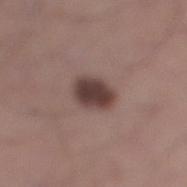Clinical impression: This lesion was catalogued during total-body skin photography and was not selected for biopsy. Image and clinical context: Longest diameter approximately 3.5 mm. Captured under white-light illumination. Cropped from a total-body skin-imaging series; the visible field is about 15 mm. A male patient in their mid-30s. Located on the leg.